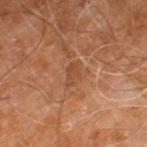<case>
<biopsy_status>not biopsied; imaged during a skin examination</biopsy_status>
<site>right leg</site>
<patient>
  <sex>male</sex>
  <age_approx>60</age_approx>
</patient>
<image>
  <source>total-body photography crop</source>
  <field_of_view_mm>15</field_of_view_mm>
</image>
</case>